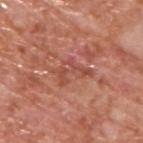{
  "biopsy_status": "not biopsied; imaged during a skin examination",
  "lesion_size": {
    "long_diameter_mm_approx": 4.5
  },
  "site": "upper back",
  "image": {
    "source": "total-body photography crop",
    "field_of_view_mm": 15
  },
  "automated_metrics": {
    "cielab_L": 50,
    "cielab_a": 28,
    "cielab_b": 30,
    "vs_skin_darker_L": 7.0,
    "vs_skin_contrast_norm": 5.5,
    "nevus_likeness_0_100": 0
  },
  "patient": {
    "sex": "male",
    "age_approx": 65
  }
}